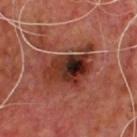Q: Was a biopsy performed?
A: no biopsy performed (imaged during a skin exam)
Q: What is the imaging modality?
A: 15 mm crop, total-body photography
Q: Who is the patient?
A: male, aged around 60
Q: Where on the body is the lesion?
A: the back
Q: Illumination type?
A: cross-polarized illumination
Q: What did automated image analysis measure?
A: a lesion area of about 20 mm², an eccentricity of roughly 0.75, and a shape-asymmetry score of about 0.35 (0 = symmetric); border irregularity of about 6 on a 0–10 scale, a color-variation rating of about 10/10, and peripheral color asymmetry of about 4; a classifier nevus-likeness of about 0/100